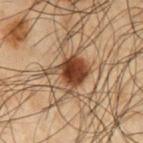Assessment:
Recorded during total-body skin imaging; not selected for excision or biopsy.